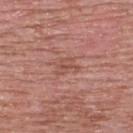The lesion is located on the upper back. A male patient, aged around 50. Measured at roughly 3 mm in maximum diameter. A lesion tile, about 15 mm wide, cut from a 3D total-body photograph.